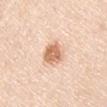biopsy status=no biopsy performed (imaged during a skin exam).